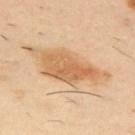Assessment:
Captured during whole-body skin photography for melanoma surveillance; the lesion was not biopsied.
Image and clinical context:
A roughly 15 mm field-of-view crop from a total-body skin photograph. Captured under cross-polarized illumination. About 10 mm across. The subject is a male aged around 40. The lesion is on the upper back. Automated image analysis of the tile measured an average lesion color of about L≈62 a*≈19 b*≈38 (CIELAB). And it measured border irregularity of about 5.5 on a 0–10 scale, a within-lesion color-variation index near 5/10, and a peripheral color-asymmetry measure near 2. It also reported a classifier nevus-likeness of about 50/100 and a detector confidence of about 100 out of 100 that the crop contains a lesion.